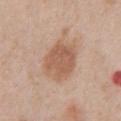No biopsy was performed on this lesion — it was imaged during a full skin examination and was not determined to be concerning. A male subject aged approximately 60. Located on the chest. A roughly 15 mm field-of-view crop from a total-body skin photograph. Automated image analysis of the tile measured a border-irregularity index near 1.5/10, internal color variation of about 3 on a 0–10 scale, and a peripheral color-asymmetry measure near 1. Captured under white-light illumination. The recorded lesion diameter is about 5.5 mm.